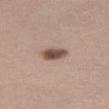Impression:
Imaged during a routine full-body skin examination; the lesion was not biopsied and no histopathology is available.
Image and clinical context:
About 3 mm across. A roughly 15 mm field-of-view crop from a total-body skin photograph. Automated image analysis of the tile measured an average lesion color of about L≈50 a*≈17 b*≈23 (CIELAB), about 15 CIELAB-L* units darker than the surrounding skin, and a normalized lesion–skin contrast near 10.5. And it measured border irregularity of about 2 on a 0–10 scale, a within-lesion color-variation index near 4/10, and radial color variation of about 1. The software also gave a nevus-likeness score of about 95/100. The lesion is on the left thigh. A female patient approximately 25 years of age. Imaged with white-light lighting.